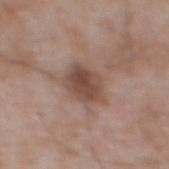<case>
<biopsy_status>not biopsied; imaged during a skin examination</biopsy_status>
<image>
  <source>total-body photography crop</source>
  <field_of_view_mm>15</field_of_view_mm>
</image>
<lighting>white-light</lighting>
<lesion_size>
  <long_diameter_mm_approx>4.0</long_diameter_mm_approx>
</lesion_size>
<patient>
  <sex>male</sex>
  <age_approx>50</age_approx>
</patient>
<site>mid back</site>
</case>